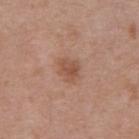biopsy status: imaged on a skin check; not biopsied | size: ~2.5 mm (longest diameter) | subject: male, aged around 70 | acquisition: 15 mm crop, total-body photography | lighting: white-light | body site: the abdomen.